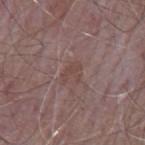Clinical impression:
No biopsy was performed on this lesion — it was imaged during a full skin examination and was not determined to be concerning.
Context:
From the mid back. This is a white-light tile. The patient is a male aged 63–67. A roughly 15 mm field-of-view crop from a total-body skin photograph. The lesion's longest dimension is about 2.5 mm.